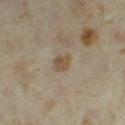The lesion was tiled from a total-body skin photograph and was not biopsied. The lesion is on the left thigh. Cropped from a total-body skin-imaging series; the visible field is about 15 mm. A female subject, roughly 35 years of age. This is a cross-polarized tile.